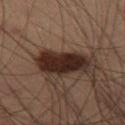Q: Is there a histopathology result?
A: total-body-photography surveillance lesion; no biopsy
Q: Who is the patient?
A: male, aged around 55
Q: How was this image acquired?
A: 15 mm crop, total-body photography
Q: What lighting was used for the tile?
A: cross-polarized
Q: Lesion location?
A: the left thigh
Q: Lesion size?
A: about 5.5 mm
Q: What did automated image analysis measure?
A: an area of roughly 15 mm², an eccentricity of roughly 0.85, and a symmetry-axis asymmetry near 0.2; a mean CIELAB color near L≈19 a*≈14 b*≈17, a lesion–skin lightness drop of about 13, and a lesion-to-skin contrast of about 15 (normalized; higher = more distinct); border irregularity of about 2.5 on a 0–10 scale